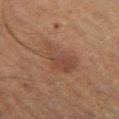The lesion was tiled from a total-body skin photograph and was not biopsied. The total-body-photography lesion software estimated a lesion area of about 13 mm², an outline eccentricity of about 0.75 (0 = round, 1 = elongated), and a shape-asymmetry score of about 0.35 (0 = symmetric). The software also gave internal color variation of about 3.5 on a 0–10 scale and radial color variation of about 1. Captured under cross-polarized illumination. A male subject, aged approximately 55. Longest diameter approximately 5.5 mm. Located on the left thigh. A 15 mm crop from a total-body photograph taken for skin-cancer surveillance.